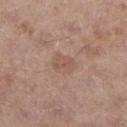Part of a total-body skin-imaging series; this lesion was reviewed on a skin check and was not flagged for biopsy.
The lesion is located on the left lower leg.
About 3 mm across.
A male subject, approximately 55 years of age.
Imaged with white-light lighting.
An algorithmic analysis of the crop reported an automated nevus-likeness rating near 0 out of 100.
A lesion tile, about 15 mm wide, cut from a 3D total-body photograph.